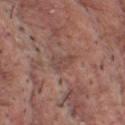follow-up: imaged on a skin check; not biopsied
image source: ~15 mm tile from a whole-body skin photo
subject: male, aged approximately 60
body site: the chest